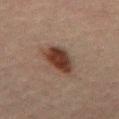Assessment:
Imaged during a routine full-body skin examination; the lesion was not biopsied and no histopathology is available.
Context:
Captured under cross-polarized illumination. The subject is a male aged 48–52. From the abdomen. A lesion tile, about 15 mm wide, cut from a 3D total-body photograph. The recorded lesion diameter is about 4 mm.